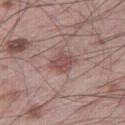workup: catalogued during a skin exam; not biopsied
automated lesion analysis: about 10 CIELAB-L* units darker than the surrounding skin
patient: male, aged 58–62
site: the right thigh
acquisition: ~15 mm tile from a whole-body skin photo